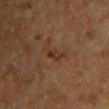notes: total-body-photography surveillance lesion; no biopsy | lesion size: ≈3 mm | subject: female, about 60 years old | image: total-body-photography crop, ~15 mm field of view | automated metrics: a lesion area of about 3 mm² and a symmetry-axis asymmetry near 0.4; a lesion color around L≈29 a*≈16 b*≈27 in CIELAB, roughly 6 lightness units darker than nearby skin, and a normalized border contrast of about 6.5 | location: the front of the torso.